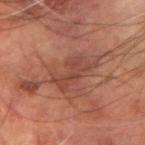automated metrics: border irregularity of about 6 on a 0–10 scale, a within-lesion color-variation index near 3/10, and a peripheral color-asymmetry measure near 1; an automated nevus-likeness rating near 0 out of 100
diameter: ≈5 mm
imaging modality: ~15 mm crop, total-body skin-cancer survey
subject: male, approximately 60 years of age
tile lighting: cross-polarized illumination
body site: the left arm